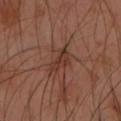Assessment: The lesion was photographed on a routine skin check and not biopsied; there is no pathology result. Context: The tile uses cross-polarized illumination. From the left forearm. Automated tile analysis of the lesion measured a lesion area of about 6.5 mm², an outline eccentricity of about 0.85 (0 = round, 1 = elongated), and a shape-asymmetry score of about 0.35 (0 = symmetric). The software also gave border irregularity of about 4 on a 0–10 scale, internal color variation of about 3.5 on a 0–10 scale, and radial color variation of about 1.5. Cropped from a whole-body photographic skin survey; the tile spans about 15 mm. Measured at roughly 4 mm in maximum diameter. The patient is a female in their mid- to late 50s.